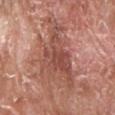The subject is a male aged around 65.
A lesion tile, about 15 mm wide, cut from a 3D total-body photograph.
The lesion is on the head or neck.